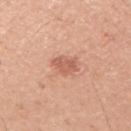Assessment: Part of a total-body skin-imaging series; this lesion was reviewed on a skin check and was not flagged for biopsy. Context: A male patient, aged around 45. On the right upper arm. A close-up tile cropped from a whole-body skin photograph, about 15 mm across. An algorithmic analysis of the crop reported a symmetry-axis asymmetry near 0.25. The analysis additionally found a lesion color around L≈60 a*≈26 b*≈31 in CIELAB, a lesion–skin lightness drop of about 10, and a lesion-to-skin contrast of about 6 (normalized; higher = more distinct). The analysis additionally found a border-irregularity rating of about 2.5/10, internal color variation of about 2 on a 0–10 scale, and a peripheral color-asymmetry measure near 0.5. This is a white-light tile.